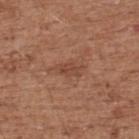| field | value |
|---|---|
| biopsy status | total-body-photography surveillance lesion; no biopsy |
| site | the back |
| lesion size | about 3 mm |
| subject | male, aged approximately 75 |
| image | total-body-photography crop, ~15 mm field of view |